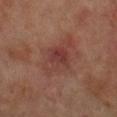biopsy status = total-body-photography surveillance lesion; no biopsy.